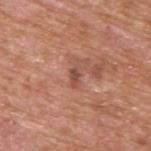biopsy status = catalogued during a skin exam; not biopsied
automated metrics = border irregularity of about 4.5 on a 0–10 scale and peripheral color asymmetry of about 0
location = the upper back
subject = male, aged 63 to 67
size = ~2.5 mm (longest diameter)
image = total-body-photography crop, ~15 mm field of view